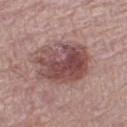The lesion was tiled from a total-body skin photograph and was not biopsied.
The subject is a female aged 63 to 67.
A 15 mm crop from a total-body photograph taken for skin-cancer surveillance.
Automated image analysis of the tile measured a mean CIELAB color near L≈48 a*≈22 b*≈20 and roughly 13 lightness units darker than nearby skin. The analysis additionally found a border-irregularity rating of about 2.5/10. And it measured an automated nevus-likeness rating near 80 out of 100.
The recorded lesion diameter is about 6 mm.
Imaged with white-light lighting.
On the left thigh.